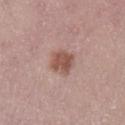  biopsy_status: not biopsied; imaged during a skin examination